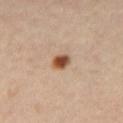Acquisition and patient details:
A region of skin cropped from a whole-body photographic capture, roughly 15 mm wide. Imaged with cross-polarized lighting. The subject is a female approximately 40 years of age. Longest diameter approximately 2 mm. On the right thigh. An algorithmic analysis of the crop reported a footprint of about 4 mm² and a symmetry-axis asymmetry near 0.15. And it measured an average lesion color of about L≈51 a*≈21 b*≈33 (CIELAB), roughly 16 lightness units darker than nearby skin, and a normalized lesion–skin contrast near 11.5.